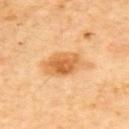Imaged during a routine full-body skin examination; the lesion was not biopsied and no histopathology is available. Captured under cross-polarized illumination. From the upper back. The subject is a male about 65 years old. Cropped from a total-body skin-imaging series; the visible field is about 15 mm. The total-body-photography lesion software estimated a lesion area of about 13 mm², a shape eccentricity near 0.8, and a symmetry-axis asymmetry near 0.2. The software also gave an average lesion color of about L≈66 a*≈25 b*≈46 (CIELAB) and roughly 12 lightness units darker than nearby skin. The analysis additionally found border irregularity of about 2.5 on a 0–10 scale, a within-lesion color-variation index near 5.5/10, and a peripheral color-asymmetry measure near 1.5. And it measured a classifier nevus-likeness of about 65/100 and a lesion-detection confidence of about 100/100. The lesion's longest dimension is about 5.5 mm.